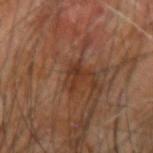<tbp_lesion>
  <biopsy_status>not biopsied; imaged during a skin examination</biopsy_status>
  <lesion_size>
    <long_diameter_mm_approx>3.5</long_diameter_mm_approx>
  </lesion_size>
  <lighting>cross-polarized</lighting>
  <patient>
    <sex>male</sex>
    <age_approx>65</age_approx>
  </patient>
  <automated_metrics>
    <area_mm2_approx>5.0</area_mm2_approx>
    <shape_asymmetry>0.4</shape_asymmetry>
    <vs_skin_contrast_norm>6.5</vs_skin_contrast_norm>
    <border_irregularity_0_10>4.5</border_irregularity_0_10>
    <color_variation_0_10>3.0</color_variation_0_10>
    <peripheral_color_asymmetry>1.0</peripheral_color_asymmetry>
  </automated_metrics>
  <image>
    <source>total-body photography crop</source>
    <field_of_view_mm>15</field_of_view_mm>
  </image>
  <site>right forearm</site>
</tbp_lesion>